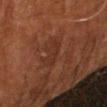• notes: imaged on a skin check; not biopsied
• subject: female, aged around 80
• acquisition: total-body-photography crop, ~15 mm field of view
• lighting: cross-polarized illumination
• TBP lesion metrics: a border-irregularity index near 6.5/10 and peripheral color asymmetry of about 0; a lesion-detection confidence of about 75/100
• anatomic site: the arm